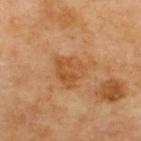{
  "biopsy_status": "not biopsied; imaged during a skin examination",
  "site": "upper back",
  "image": {
    "source": "total-body photography crop",
    "field_of_view_mm": 15
  },
  "lesion_size": {
    "long_diameter_mm_approx": 4.5
  }
}